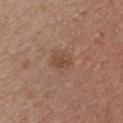This lesion was catalogued during total-body skin photography and was not selected for biopsy. A roughly 15 mm field-of-view crop from a total-body skin photograph. The lesion-visualizer software estimated a footprint of about 4 mm² and a shape eccentricity near 0.65. It also reported a lesion–skin lightness drop of about 7 and a normalized lesion–skin contrast near 6. The analysis additionally found a border-irregularity rating of about 1.5/10 and a within-lesion color-variation index near 1.5/10. The software also gave an automated nevus-likeness rating near 5 out of 100 and a lesion-detection confidence of about 100/100. The recorded lesion diameter is about 2.5 mm. A female subject aged approximately 30. Imaged with white-light lighting. On the chest.